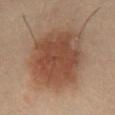notes: no biopsy performed (imaged during a skin exam)
location: the abdomen
imaging modality: ~15 mm tile from a whole-body skin photo
illumination: cross-polarized
diameter: about 10 mm
image-analysis metrics: a lesion area of about 48 mm² and a shape-asymmetry score of about 0.1 (0 = symmetric); a nevus-likeness score of about 100/100 and a lesion-detection confidence of about 100/100
patient: male, aged approximately 40